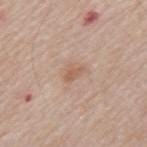Impression: Part of a total-body skin-imaging series; this lesion was reviewed on a skin check and was not flagged for biopsy. Acquisition and patient details: A male patient, about 65 years old. The lesion is on the mid back. A 15 mm crop from a total-body photograph taken for skin-cancer surveillance. Imaged with white-light lighting. The lesion's longest dimension is about 2.5 mm.